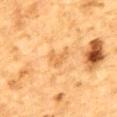Impression: Imaged during a routine full-body skin examination; the lesion was not biopsied and no histopathology is available. Acquisition and patient details: The subject is a male aged approximately 85 or older. An algorithmic analysis of the crop reported an outline eccentricity of about 0.75 (0 = round, 1 = elongated) and a shape-asymmetry score of about 0.5 (0 = symmetric). The software also gave an average lesion color of about L≈59 a*≈21 b*≈43 (CIELAB) and a lesion-to-skin contrast of about 5 (normalized; higher = more distinct). It also reported a within-lesion color-variation index near 2/10 and peripheral color asymmetry of about 0.5. The lesion is on the mid back. Approximately 3 mm at its widest. A 15 mm close-up tile from a total-body photography series done for melanoma screening.